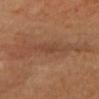notes=total-body-photography surveillance lesion; no biopsy
patient=female, in their 70s
site=the head or neck
tile lighting=cross-polarized illumination
image=~15 mm crop, total-body skin-cancer survey
lesion diameter=about 2.5 mm
TBP lesion metrics=a lesion area of about 2 mm² and two-axis asymmetry of about 0.5; a border-irregularity index near 5/10, a within-lesion color-variation index near 0/10, and peripheral color asymmetry of about 0; an automated nevus-likeness rating near 0 out of 100 and a lesion-detection confidence of about 85/100